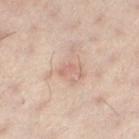Q: Is there a histopathology result?
A: catalogued during a skin exam; not biopsied
Q: What lighting was used for the tile?
A: cross-polarized illumination
Q: Where on the body is the lesion?
A: the left lower leg
Q: Automated lesion metrics?
A: a lesion area of about 2.5 mm², an eccentricity of roughly 0.9, and a symmetry-axis asymmetry near 0.5; a border-irregularity index near 4.5/10, a within-lesion color-variation index near 0/10, and a peripheral color-asymmetry measure near 0
Q: What is the lesion's diameter?
A: ~2.5 mm (longest diameter)
Q: What is the imaging modality?
A: 15 mm crop, total-body photography
Q: What are the patient's age and sex?
A: female, roughly 30 years of age